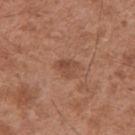Impression: Recorded during total-body skin imaging; not selected for excision or biopsy. Acquisition and patient details: Cropped from a total-body skin-imaging series; the visible field is about 15 mm. The patient is a male aged 48 to 52. The lesion is on the right upper arm. The lesion's longest dimension is about 2.5 mm.